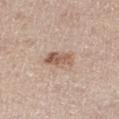<lesion>
<biopsy_status>not biopsied; imaged during a skin examination</biopsy_status>
<lighting>white-light</lighting>
<automated_metrics>
  <area_mm2_approx>6.5</area_mm2_approx>
  <shape_asymmetry>0.25</shape_asymmetry>
  <cielab_L>58</cielab_L>
  <cielab_a>18</cielab_a>
  <cielab_b>28</cielab_b>
  <vs_skin_darker_L>11.0</vs_skin_darker_L>
  <border_irregularity_0_10>3.0</border_irregularity_0_10>
  <peripheral_color_asymmetry>2.0</peripheral_color_asymmetry>
</automated_metrics>
<image>
  <source>total-body photography crop</source>
  <field_of_view_mm>15</field_of_view_mm>
</image>
<patient>
  <sex>female</sex>
  <age_approx>50</age_approx>
</patient>
<lesion_size>
  <long_diameter_mm_approx>4.0</long_diameter_mm_approx>
</lesion_size>
<site>right thigh</site>
</lesion>